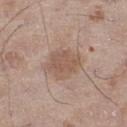Impression: No biopsy was performed on this lesion — it was imaged during a full skin examination and was not determined to be concerning. Background: Measured at roughly 4 mm in maximum diameter. This is a white-light tile. The lesion is located on the left thigh. The patient is a male aged around 65. An algorithmic analysis of the crop reported a mean CIELAB color near L≈54 a*≈17 b*≈27 and a lesion–skin lightness drop of about 8. Cropped from a total-body skin-imaging series; the visible field is about 15 mm.